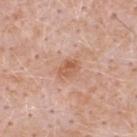<case>
<biopsy_status>not biopsied; imaged during a skin examination</biopsy_status>
<lighting>white-light</lighting>
<lesion_size>
  <long_diameter_mm_approx>3.0</long_diameter_mm_approx>
</lesion_size>
<automated_metrics>
  <color_variation_0_10>4.0</color_variation_0_10>
  <peripheral_color_asymmetry>1.5</peripheral_color_asymmetry>
  <nevus_likeness_0_100>10</nevus_likeness_0_100>
  <lesion_detection_confidence_0_100>100</lesion_detection_confidence_0_100>
</automated_metrics>
<site>upper back</site>
<image>
  <source>total-body photography crop</source>
  <field_of_view_mm>15</field_of_view_mm>
</image>
<patient>
  <sex>male</sex>
  <age_approx>50</age_approx>
</patient>
</case>